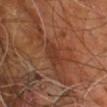Recorded during total-body skin imaging; not selected for excision or biopsy. Located on the leg. The recorded lesion diameter is about 3 mm. A lesion tile, about 15 mm wide, cut from a 3D total-body photograph. The lesion-visualizer software estimated a lesion area of about 5.5 mm², an eccentricity of roughly 0.75, and a symmetry-axis asymmetry near 0.25. It also reported a mean CIELAB color near L≈34 a*≈22 b*≈29 and roughly 6 lightness units darker than nearby skin. A male patient, aged approximately 60. Imaged with cross-polarized lighting.